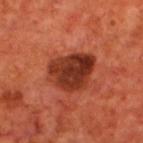notes=total-body-photography surveillance lesion; no biopsy | subject=male, aged around 70 | lesion diameter=~5.5 mm (longest diameter) | site=the upper back | illumination=cross-polarized illumination | automated metrics=a lesion area of about 18 mm², an eccentricity of roughly 0.6, and two-axis asymmetry of about 0.25; a color-variation rating of about 6.5/10 | acquisition=~15 mm tile from a whole-body skin photo.